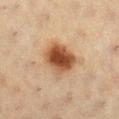Q: Was this lesion biopsied?
A: catalogued during a skin exam; not biopsied
Q: Where on the body is the lesion?
A: the right lower leg
Q: What did automated image analysis measure?
A: an outline eccentricity of about 0.55 (0 = round, 1 = elongated) and a shape-asymmetry score of about 0.2 (0 = symmetric); a mean CIELAB color near L≈44 a*≈19 b*≈31, about 15 CIELAB-L* units darker than the surrounding skin, and a lesion-to-skin contrast of about 11 (normalized; higher = more distinct); a border-irregularity rating of about 2/10, internal color variation of about 7 on a 0–10 scale, and a peripheral color-asymmetry measure near 2; a classifier nevus-likeness of about 100/100 and a lesion-detection confidence of about 100/100
Q: How large is the lesion?
A: about 4.5 mm
Q: Patient demographics?
A: female, in their 40s
Q: How was this image acquired?
A: 15 mm crop, total-body photography